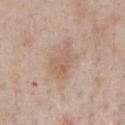notes: total-body-photography surveillance lesion; no biopsy
body site: the chest
patient: male, approximately 60 years of age
illumination: white-light
acquisition: total-body-photography crop, ~15 mm field of view